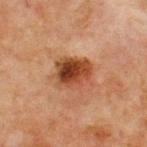No biopsy was performed on this lesion — it was imaged during a full skin examination and was not determined to be concerning.
Imaged with cross-polarized lighting.
Cropped from a total-body skin-imaging series; the visible field is about 15 mm.
The subject is a male roughly 65 years of age.
The lesion's longest dimension is about 4 mm.
The lesion is on the chest.